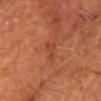workup = catalogued during a skin exam; not biopsied
body site = the head or neck
image source = 15 mm crop, total-body photography
TBP lesion metrics = border irregularity of about 5.5 on a 0–10 scale and a peripheral color-asymmetry measure near 0
subject = male, approximately 65 years of age